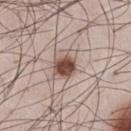The lesion's longest dimension is about 3.5 mm.
A male patient, about 35 years old.
The total-body-photography lesion software estimated a lesion color around L≈51 a*≈18 b*≈24 in CIELAB.
From the abdomen.
This is a white-light tile.
A lesion tile, about 15 mm wide, cut from a 3D total-body photograph.